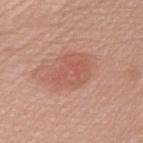Assessment:
Recorded during total-body skin imaging; not selected for excision or biopsy.
Clinical summary:
This image is a 15 mm lesion crop taken from a total-body photograph. The tile uses white-light illumination. Automated tile analysis of the lesion measured a mean CIELAB color near L≈57 a*≈26 b*≈29, about 7 CIELAB-L* units darker than the surrounding skin, and a lesion-to-skin contrast of about 5 (normalized; higher = more distinct). The software also gave a color-variation rating of about 3/10 and radial color variation of about 1. From the right upper arm. Approximately 4.5 mm at its widest. The patient is a female in their mid- to late 60s.